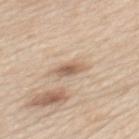Assessment: This lesion was catalogued during total-body skin photography and was not selected for biopsy. Clinical summary: Automated image analysis of the tile measured an average lesion color of about L≈60 a*≈17 b*≈29 (CIELAB), about 12 CIELAB-L* units darker than the surrounding skin, and a normalized lesion–skin contrast near 7.5. Captured under white-light illumination. The patient is a male aged 58–62. Cropped from a whole-body photographic skin survey; the tile spans about 15 mm. The lesion is on the mid back.